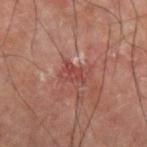Assessment: Imaged during a routine full-body skin examination; the lesion was not biopsied and no histopathology is available. Image and clinical context: The subject is a male aged 58–62. A close-up tile cropped from a whole-body skin photograph, about 15 mm across. The lesion is on the left forearm.